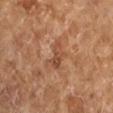workup = total-body-photography surveillance lesion; no biopsy
site = the right forearm
lesion size = about 3.5 mm
patient = female, roughly 70 years of age
automated metrics = a border-irregularity index near 5/10 and peripheral color asymmetry of about 2.5
image source = 15 mm crop, total-body photography
illumination = cross-polarized